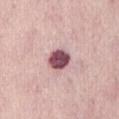<case>
  <biopsy_status>not biopsied; imaged during a skin examination</biopsy_status>
  <image>
    <source>total-body photography crop</source>
    <field_of_view_mm>15</field_of_view_mm>
  </image>
  <lesion_size>
    <long_diameter_mm_approx>3.0</long_diameter_mm_approx>
  </lesion_size>
  <site>abdomen</site>
  <patient>
    <sex>male</sex>
    <age_approx>85+</age_approx>
  </patient>
  <lighting>white-light</lighting>
</case>